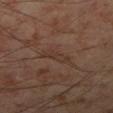Imaged during a routine full-body skin examination; the lesion was not biopsied and no histopathology is available. This is a cross-polarized tile. About 4 mm across. Located on the right lower leg. The patient is a male in their mid-50s. Cropped from a whole-body photographic skin survey; the tile spans about 15 mm. The total-body-photography lesion software estimated a lesion area of about 4.5 mm² and two-axis asymmetry of about 0.35. The software also gave a mean CIELAB color near L≈34 a*≈16 b*≈23, roughly 5 lightness units darker than nearby skin, and a normalized lesion–skin contrast near 5. The analysis additionally found border irregularity of about 4.5 on a 0–10 scale, a color-variation rating of about 0.5/10, and peripheral color asymmetry of about 0. It also reported a nevus-likeness score of about 0/100 and a lesion-detection confidence of about 85/100.